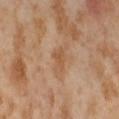biopsy_status: not biopsied; imaged during a skin examination
lesion_size:
  long_diameter_mm_approx: 3.0
lighting: cross-polarized
automated_metrics:
  cielab_L: 55
  cielab_a: 20
  cielab_b: 35
  vs_skin_darker_L: 7.0
  vs_skin_contrast_norm: 5.5
site: right thigh
patient:
  sex: female
  age_approx: 55
image:
  source: total-body photography crop
  field_of_view_mm: 15